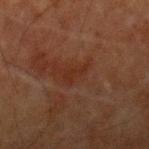Captured during whole-body skin photography for melanoma surveillance; the lesion was not biopsied.